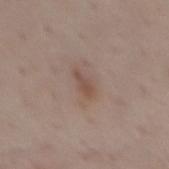Assessment:
This lesion was catalogued during total-body skin photography and was not selected for biopsy.
Context:
A roughly 15 mm field-of-view crop from a total-body skin photograph. This is a white-light tile. The lesion's longest dimension is about 3 mm. A female subject roughly 45 years of age. The lesion is located on the back.